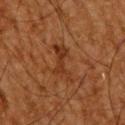The lesion was tiled from a total-body skin photograph and was not biopsied.
The lesion is on the back.
An algorithmic analysis of the crop reported an average lesion color of about L≈28 a*≈20 b*≈29 (CIELAB), about 6 CIELAB-L* units darker than the surrounding skin, and a normalized border contrast of about 6.5. And it measured a border-irregularity index near 7/10, a within-lesion color-variation index near 2/10, and a peripheral color-asymmetry measure near 0.5. The software also gave lesion-presence confidence of about 100/100.
The subject is a male roughly 65 years of age.
The tile uses cross-polarized illumination.
Measured at roughly 5 mm in maximum diameter.
Cropped from a total-body skin-imaging series; the visible field is about 15 mm.